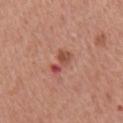Imaged during a routine full-body skin examination; the lesion was not biopsied and no histopathology is available.
An algorithmic analysis of the crop reported a border-irregularity index near 5/10, internal color variation of about 2.5 on a 0–10 scale, and peripheral color asymmetry of about 1.
This image is a 15 mm lesion crop taken from a total-body photograph.
A male patient in their mid- to late 60s.
The lesion's longest dimension is about 3 mm.
Located on the front of the torso.
The tile uses white-light illumination.